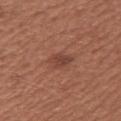Captured during whole-body skin photography for melanoma surveillance; the lesion was not biopsied.
A roughly 15 mm field-of-view crop from a total-body skin photograph.
An algorithmic analysis of the crop reported a mean CIELAB color near L≈42 a*≈24 b*≈27, a lesion–skin lightness drop of about 8, and a lesion-to-skin contrast of about 6.5 (normalized; higher = more distinct). The analysis additionally found a color-variation rating of about 2/10.
The patient is a female roughly 55 years of age.
The lesion is on the left upper arm.
About 2.5 mm across.
The tile uses white-light illumination.